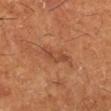Image and clinical context: The lesion is on the leg. A 15 mm crop from a total-body photograph taken for skin-cancer surveillance. A male patient, aged 63 to 67.